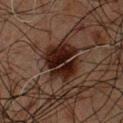This lesion was catalogued during total-body skin photography and was not selected for biopsy. A roughly 15 mm field-of-view crop from a total-body skin photograph. Captured under cross-polarized illumination. Automated tile analysis of the lesion measured a shape eccentricity near 0.5 and a shape-asymmetry score of about 0.25 (0 = symmetric). It also reported an average lesion color of about L≈16 a*≈15 b*≈17 (CIELAB), a lesion–skin lightness drop of about 11, and a normalized border contrast of about 14. And it measured a nevus-likeness score of about 85/100 and lesion-presence confidence of about 100/100. The subject is a male aged 58 to 62. On the chest.